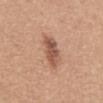  biopsy_status: not biopsied; imaged during a skin examination
  automated_metrics:
    border_irregularity_0_10: 2.5
    color_variation_0_10: 4.0
    peripheral_color_asymmetry: 1.5
    nevus_likeness_0_100: 85
    lesion_detection_confidence_0_100: 100
  lesion_size:
    long_diameter_mm_approx: 4.5
  patient:
    sex: female
    age_approx: 40
  image:
    source: total-body photography crop
    field_of_view_mm: 15
  lighting: white-light
  site: abdomen